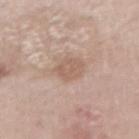The lesion was tiled from a total-body skin photograph and was not biopsied. A roughly 15 mm field-of-view crop from a total-body skin photograph. The lesion is on the right upper arm. About 3 mm across. A male patient in their 60s.